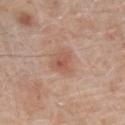follow-up=total-body-photography surveillance lesion; no biopsy | automated metrics=a border-irregularity rating of about 3.5/10, internal color variation of about 4.5 on a 0–10 scale, and peripheral color asymmetry of about 1.5; a nevus-likeness score of about 30/100 and lesion-presence confidence of about 100/100 | patient=male, about 70 years old | location=the left lower leg | imaging modality=15 mm crop, total-body photography | tile lighting=white-light.